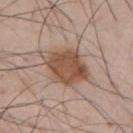The lesion was photographed on a routine skin check and not biopsied; there is no pathology result. The subject is a male approximately 55 years of age. The lesion's longest dimension is about 6 mm. A 15 mm crop from a total-body photograph taken for skin-cancer surveillance. The lesion is located on the upper back. The total-body-photography lesion software estimated an area of roughly 18 mm² and two-axis asymmetry of about 0.25. It also reported a classifier nevus-likeness of about 90/100. Imaged with white-light lighting.